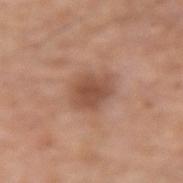<tbp_lesion>
  <biopsy_status>not biopsied; imaged during a skin examination</biopsy_status>
  <site>right forearm</site>
  <lesion_size>
    <long_diameter_mm_approx>4.0</long_diameter_mm_approx>
  </lesion_size>
  <image>
    <source>total-body photography crop</source>
    <field_of_view_mm>15</field_of_view_mm>
  </image>
  <patient>
    <sex>male</sex>
    <age_approx>80</age_approx>
  </patient>
</tbp_lesion>